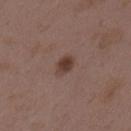biopsy status: imaged on a skin check; not biopsied
location: the back
diameter: ≈2.5 mm
imaging modality: 15 mm crop, total-body photography
subject: female, about 35 years old
lighting: white-light